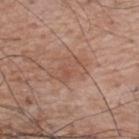Q: What is the anatomic site?
A: the upper back
Q: Who is the patient?
A: male, roughly 70 years of age
Q: How was this image acquired?
A: ~15 mm crop, total-body skin-cancer survey
Q: How large is the lesion?
A: about 4 mm
Q: What lighting was used for the tile?
A: white-light illumination